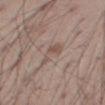Q: Was a biopsy performed?
A: total-body-photography surveillance lesion; no biopsy